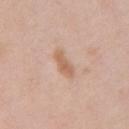A 15 mm crop from a total-body photograph taken for skin-cancer surveillance.
The tile uses white-light illumination.
On the front of the torso.
About 3.5 mm across.
A female subject aged around 50.
Automated tile analysis of the lesion measured a lesion area of about 4.5 mm², a shape eccentricity near 0.9, and a shape-asymmetry score of about 0.3 (0 = symmetric). It also reported a border-irregularity rating of about 3/10, a within-lesion color-variation index near 2/10, and radial color variation of about 0.5.